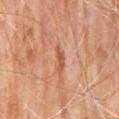Q: Was a biopsy performed?
A: no biopsy performed (imaged during a skin exam)
Q: Where on the body is the lesion?
A: the mid back
Q: How was this image acquired?
A: ~15 mm tile from a whole-body skin photo
Q: Who is the patient?
A: male, in their 70s
Q: Lesion size?
A: about 3.5 mm